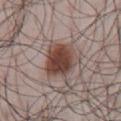Assessment:
Part of a total-body skin-imaging series; this lesion was reviewed on a skin check and was not flagged for biopsy.
Context:
The lesion is on the abdomen. A 15 mm crop from a total-body photograph taken for skin-cancer surveillance. A male subject, roughly 45 years of age.